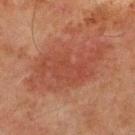Q: Was this lesion biopsied?
A: total-body-photography surveillance lesion; no biopsy
Q: How was this image acquired?
A: total-body-photography crop, ~15 mm field of view
Q: Lesion location?
A: the front of the torso
Q: Automated lesion metrics?
A: a footprint of about 31 mm²; an average lesion color of about L≈37 a*≈23 b*≈26 (CIELAB), a lesion–skin lightness drop of about 6, and a normalized lesion–skin contrast near 5.5; a border-irregularity index near 4.5/10, a within-lesion color-variation index near 3/10, and radial color variation of about 1
Q: Patient demographics?
A: male, aged around 65
Q: What lighting was used for the tile?
A: cross-polarized